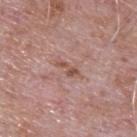Case summary:
– biopsy status: catalogued during a skin exam; not biopsied
– size: ≈3 mm
– image-analysis metrics: an eccentricity of roughly 0.9 and two-axis asymmetry of about 0.45; a border-irregularity rating of about 5.5/10, internal color variation of about 0 on a 0–10 scale, and a peripheral color-asymmetry measure near 0
– image source: ~15 mm crop, total-body skin-cancer survey
– patient: male, roughly 65 years of age
– body site: the back